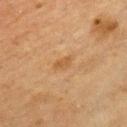Context: An algorithmic analysis of the crop reported a lesion area of about 3 mm², an outline eccentricity of about 0.85 (0 = round, 1 = elongated), and two-axis asymmetry of about 0.3. The software also gave an average lesion color of about L≈45 a*≈17 b*≈34 (CIELAB). A male patient, in their mid-40s. A 15 mm crop from a total-body photograph taken for skin-cancer surveillance. Longest diameter approximately 2.5 mm. The lesion is on the chest.